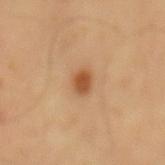This lesion was catalogued during total-body skin photography and was not selected for biopsy.